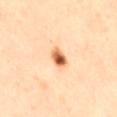<tbp_lesion>
  <biopsy_status>not biopsied; imaged during a skin examination</biopsy_status>
  <automated_metrics>
    <area_mm2_approx>4.0</area_mm2_approx>
    <eccentricity>0.65</eccentricity>
    <shape_asymmetry>0.2</shape_asymmetry>
    <cielab_L>65</cielab_L>
    <cielab_a>27</cielab_a>
    <cielab_b>42</cielab_b>
    <vs_skin_darker_L>20.0</vs_skin_darker_L>
    <border_irregularity_0_10>1.5</border_irregularity_0_10>
    <color_variation_0_10>9.0</color_variation_0_10>
    <peripheral_color_asymmetry>4.0</peripheral_color_asymmetry>
    <nevus_likeness_0_100>100</nevus_likeness_0_100>
  </automated_metrics>
  <image>
    <source>total-body photography crop</source>
    <field_of_view_mm>15</field_of_view_mm>
  </image>
  <patient>
    <sex>male</sex>
    <age_approx>50</age_approx>
  </patient>
  <lighting>cross-polarized</lighting>
  <site>right thigh</site>
</tbp_lesion>